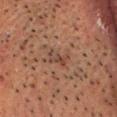Imaged during a routine full-body skin examination; the lesion was not biopsied and no histopathology is available. A 15 mm crop from a total-body photograph taken for skin-cancer surveillance. On the head or neck. A male patient, about 50 years old. Longest diameter approximately 3 mm.